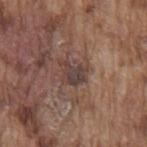workup: total-body-photography surveillance lesion; no biopsy | patient: male, aged 73–77 | imaging modality: total-body-photography crop, ~15 mm field of view | size: ~3.5 mm (longest diameter) | location: the mid back | TBP lesion metrics: a lesion area of about 6 mm² and a shape-asymmetry score of about 0.4 (0 = symmetric); a mean CIELAB color near L≈40 a*≈16 b*≈20 and roughly 9 lightness units darker than nearby skin; peripheral color asymmetry of about 1 | illumination: white-light illumination.